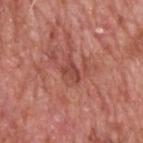notes: total-body-photography surveillance lesion; no biopsy
automated lesion analysis: an average lesion color of about L≈46 a*≈28 b*≈27 (CIELAB), about 8 CIELAB-L* units darker than the surrounding skin, and a lesion-to-skin contrast of about 6.5 (normalized; higher = more distinct)
imaging modality: 15 mm crop, total-body photography
patient: male, aged approximately 60
site: the head or neck
lighting: white-light